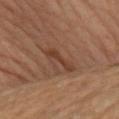Imaged with cross-polarized lighting.
Approximately 4 mm at its widest.
Automated tile analysis of the lesion measured a lesion color around L≈39 a*≈20 b*≈28 in CIELAB and a normalized lesion–skin contrast near 6.5. The software also gave a border-irregularity rating of about 4.5/10, internal color variation of about 2.5 on a 0–10 scale, and a peripheral color-asymmetry measure near 0.5. It also reported an automated nevus-likeness rating near 45 out of 100.
The lesion is on the left arm.
A female patient, aged 63 to 67.
A close-up tile cropped from a whole-body skin photograph, about 15 mm across.